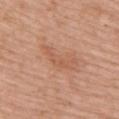Q: Was a biopsy performed?
A: no biopsy performed (imaged during a skin exam)
Q: Illumination type?
A: white-light illumination
Q: Who is the patient?
A: female, in their mid- to late 60s
Q: What is the anatomic site?
A: the right upper arm
Q: What did automated image analysis measure?
A: a footprint of about 5 mm², an eccentricity of roughly 0.95, and a shape-asymmetry score of about 0.7 (0 = symmetric)
Q: What is the imaging modality?
A: 15 mm crop, total-body photography